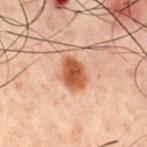Assessment: This lesion was catalogued during total-body skin photography and was not selected for biopsy. Acquisition and patient details: Automated tile analysis of the lesion measured a footprint of about 8.5 mm² and a shape-asymmetry score of about 0.2 (0 = symmetric). About 4 mm across. The tile uses cross-polarized illumination. A 15 mm close-up extracted from a 3D total-body photography capture. The lesion is on the chest. The patient is a male aged 58–62.